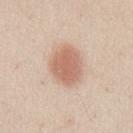follow-up: catalogued during a skin exam; not biopsied | tile lighting: white-light illumination | diameter: ≈4.5 mm | location: the chest | subject: male, in their mid- to late 20s | image source: 15 mm crop, total-body photography.